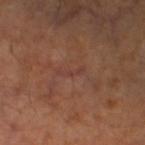<record>
<biopsy_status>not biopsied; imaged during a skin examination</biopsy_status>
<site>left lower leg</site>
<image>
  <source>total-body photography crop</source>
  <field_of_view_mm>15</field_of_view_mm>
</image>
<patient>
  <sex>male</sex>
  <age_approx>45</age_approx>
</patient>
</record>